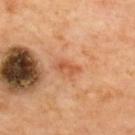No biopsy was performed on this lesion — it was imaged during a full skin examination and was not determined to be concerning. About 3 mm across. The lesion is located on the upper back. A region of skin cropped from a whole-body photographic capture, roughly 15 mm wide.